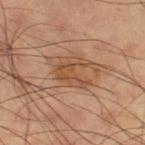biopsy_status: not biopsied; imaged during a skin examination
lesion_size:
  long_diameter_mm_approx: 4.0
automated_metrics:
  area_mm2_approx: 7.0
  eccentricity: 0.65
  shape_asymmetry: 0.65
  cielab_L: 48
  cielab_a: 21
  cielab_b: 33
  vs_skin_darker_L: 8.0
  nevus_likeness_0_100: 5
  lesion_detection_confidence_0_100: 100
image:
  source: total-body photography crop
  field_of_view_mm: 15
patient:
  sex: male
  age_approx: 60
site: right thigh
lighting: cross-polarized